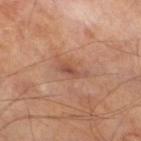Part of a total-body skin-imaging series; this lesion was reviewed on a skin check and was not flagged for biopsy.
The lesion is on the right thigh.
Measured at roughly 3.5 mm in maximum diameter.
The subject is a male aged 68 to 72.
A 15 mm close-up tile from a total-body photography series done for melanoma screening.
Imaged with cross-polarized lighting.
Automated tile analysis of the lesion measured an automated nevus-likeness rating near 0 out of 100.